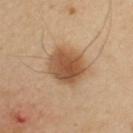| key | value |
|---|---|
| biopsy status | catalogued during a skin exam; not biopsied |
| tile lighting | cross-polarized |
| body site | the left upper arm |
| subject | female, aged 38–42 |
| lesion diameter | ≈5 mm |
| acquisition | total-body-photography crop, ~15 mm field of view |